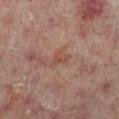* workup: catalogued during a skin exam; not biopsied
* diameter: ~2.5 mm (longest diameter)
* automated metrics: a classifier nevus-likeness of about 0/100 and lesion-presence confidence of about 100/100
* body site: the right lower leg
* subject: male, aged 63–67
* illumination: cross-polarized illumination
* image source: total-body-photography crop, ~15 mm field of view